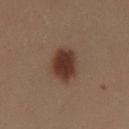Recorded during total-body skin imaging; not selected for excision or biopsy. Located on the left thigh. Cropped from a total-body skin-imaging series; the visible field is about 15 mm. A female subject, aged 28–32. Captured under cross-polarized illumination. Measured at roughly 5 mm in maximum diameter.